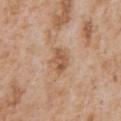The lesion was tiled from a total-body skin photograph and was not biopsied. A male subject aged 63 to 67. The lesion is on the chest. A 15 mm close-up tile from a total-body photography series done for melanoma screening.